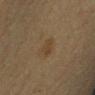The lesion was photographed on a routine skin check and not biopsied; there is no pathology result.
The lesion is located on the left forearm.
A female subject roughly 55 years of age.
Cropped from a total-body skin-imaging series; the visible field is about 15 mm.